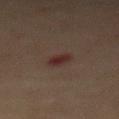Q: Was this lesion biopsied?
A: imaged on a skin check; not biopsied
Q: What is the lesion's diameter?
A: about 2.5 mm
Q: What is the imaging modality?
A: 15 mm crop, total-body photography
Q: Where on the body is the lesion?
A: the back
Q: What are the patient's age and sex?
A: female, in their 70s